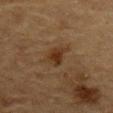Notes:
* acquisition: 15 mm crop, total-body photography
* lighting: cross-polarized
* lesion size: ≈2.5 mm
* anatomic site: the mid back
* patient: male, approximately 85 years of age or older